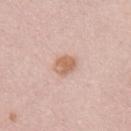- acquisition: ~15 mm crop, total-body skin-cancer survey
- TBP lesion metrics: an area of roughly 5.5 mm², an eccentricity of roughly 0.6, and a symmetry-axis asymmetry near 0.15; internal color variation of about 3 on a 0–10 scale and peripheral color asymmetry of about 1; a lesion-detection confidence of about 100/100
- lighting: white-light
- anatomic site: the right upper arm
- diameter: ≈3 mm
- patient: female, approximately 45 years of age